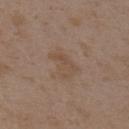Imaged during a routine full-body skin examination; the lesion was not biopsied and no histopathology is available.
A female subject about 35 years old.
From the upper back.
Cropped from a whole-body photographic skin survey; the tile spans about 15 mm.
This is a white-light tile.